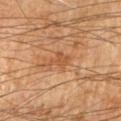– notes: no biopsy performed (imaged during a skin exam)
– lighting: cross-polarized
– image: ~15 mm crop, total-body skin-cancer survey
– patient: male, in their 70s
– image-analysis metrics: a lesion–skin lightness drop of about 7 and a lesion-to-skin contrast of about 6 (normalized; higher = more distinct); a border-irregularity index near 4/10 and peripheral color asymmetry of about 0; a nevus-likeness score of about 0/100 and a detector confidence of about 100 out of 100 that the crop contains a lesion
– body site: the right forearm
– lesion diameter: about 2.5 mm